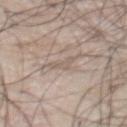Findings:
- notes · catalogued during a skin exam; not biopsied
- size · about 2.5 mm
- patient · male, aged 48–52
- location · the front of the torso
- imaging modality · total-body-photography crop, ~15 mm field of view
- lighting · white-light illumination
- image-analysis metrics · a lesion-to-skin contrast of about 4 (normalized; higher = more distinct); a border-irregularity index near 5.5/10, a color-variation rating of about 0/10, and a peripheral color-asymmetry measure near 0; a classifier nevus-likeness of about 0/100 and a detector confidence of about 0 out of 100 that the crop contains a lesion